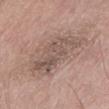Notes:
• follow-up — imaged on a skin check; not biopsied
• location — the left thigh
• subject — male, in their mid- to late 70s
• size — about 7 mm
• automated lesion analysis — a lesion color around L≈53 a*≈15 b*≈23 in CIELAB, roughly 8 lightness units darker than nearby skin, and a lesion-to-skin contrast of about 6 (normalized; higher = more distinct); a color-variation rating of about 4/10 and radial color variation of about 1.5; an automated nevus-likeness rating near 0 out of 100
• image source — ~15 mm crop, total-body skin-cancer survey